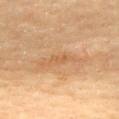Findings:
– workup: total-body-photography surveillance lesion; no biopsy
– subject: female, in their 70s
– site: the upper back
– size: about 3 mm
– illumination: cross-polarized
– image source: total-body-photography crop, ~15 mm field of view